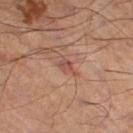Assessment:
Captured during whole-body skin photography for melanoma surveillance; the lesion was not biopsied.
Context:
Captured under cross-polarized illumination. Automated image analysis of the tile measured an average lesion color of about L≈47 a*≈22 b*≈25 (CIELAB) and a lesion-to-skin contrast of about 6 (normalized; higher = more distinct). And it measured border irregularity of about 4.5 on a 0–10 scale and a color-variation rating of about 3.5/10. The software also gave a nevus-likeness score of about 0/100 and a detector confidence of about 100 out of 100 that the crop contains a lesion. The subject is a male about 55 years old. This image is a 15 mm lesion crop taken from a total-body photograph. About 3 mm across. The lesion is located on the left lower leg.